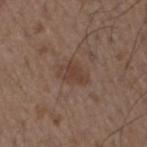Imaged during a routine full-body skin examination; the lesion was not biopsied and no histopathology is available. Located on the right upper arm. A 15 mm close-up extracted from a 3D total-body photography capture. The tile uses white-light illumination. A male patient, roughly 50 years of age.